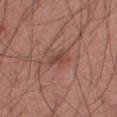Notes:
- follow-up: no biopsy performed (imaged during a skin exam)
- lighting: white-light illumination
- site: the abdomen
- size: ~3 mm (longest diameter)
- patient: male, aged 53–57
- image source: ~15 mm crop, total-body skin-cancer survey